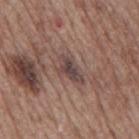<case>
<biopsy_status>not biopsied; imaged during a skin examination</biopsy_status>
<lesion_size>
  <long_diameter_mm_approx>4.5</long_diameter_mm_approx>
</lesion_size>
<patient>
  <sex>male</sex>
  <age_approx>70</age_approx>
</patient>
<image>
  <source>total-body photography crop</source>
  <field_of_view_mm>15</field_of_view_mm>
</image>
<lighting>white-light</lighting>
<site>mid back</site>
</case>